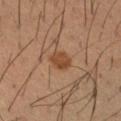Impression:
Imaged during a routine full-body skin examination; the lesion was not biopsied and no histopathology is available.